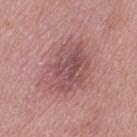Image and clinical context:
Imaged with white-light lighting. The subject is a female about 70 years old. The lesion is located on the left thigh. Longest diameter approximately 6.5 mm. A close-up tile cropped from a whole-body skin photograph, about 15 mm across. An algorithmic analysis of the crop reported an area of roughly 23 mm² and an outline eccentricity of about 0.65 (0 = round, 1 = elongated). The software also gave roughly 9 lightness units darker than nearby skin and a normalized border contrast of about 6.5.